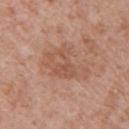follow-up = no biopsy performed (imaged during a skin exam)
image = ~15 mm crop, total-body skin-cancer survey
patient = male, aged approximately 70
location = the upper back